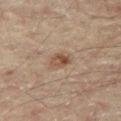Clinical impression:
The lesion was tiled from a total-body skin photograph and was not biopsied.